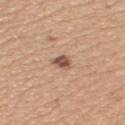No biopsy was performed on this lesion — it was imaged during a full skin examination and was not determined to be concerning. Measured at roughly 2.5 mm in maximum diameter. A female subject, aged 28 to 32. Captured under white-light illumination. The lesion is on the left upper arm. Automated tile analysis of the lesion measured a lesion area of about 3.5 mm² and a symmetry-axis asymmetry near 0.2. And it measured an average lesion color of about L≈54 a*≈19 b*≈28 (CIELAB), roughly 14 lightness units darker than nearby skin, and a lesion-to-skin contrast of about 9.5 (normalized; higher = more distinct). A 15 mm close-up extracted from a 3D total-body photography capture.